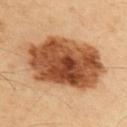Q: Is there a histopathology result?
A: no biopsy performed (imaged during a skin exam)
Q: What is the imaging modality?
A: 15 mm crop, total-body photography
Q: What is the anatomic site?
A: the upper back
Q: What is the lesion's diameter?
A: ≈11.5 mm
Q: How was the tile lit?
A: cross-polarized illumination
Q: What are the patient's age and sex?
A: male, roughly 50 years of age